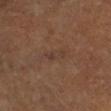workup: no biopsy performed (imaged during a skin exam) | body site: the left lower leg | lighting: cross-polarized illumination | patient: female, about 65 years old | imaging modality: ~15 mm tile from a whole-body skin photo.